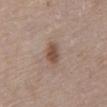<tbp_lesion>
  <biopsy_status>not biopsied; imaged during a skin examination</biopsy_status>
</tbp_lesion>